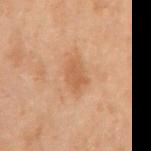| field | value |
|---|---|
| notes | imaged on a skin check; not biopsied |
| patient | male, approximately 70 years of age |
| image | ~15 mm crop, total-body skin-cancer survey |
| body site | the mid back |
| automated lesion analysis | a lesion color around L≈44 a*≈18 b*≈29 in CIELAB and roughly 6 lightness units darker than nearby skin; a border-irregularity rating of about 2.5/10, internal color variation of about 2 on a 0–10 scale, and a peripheral color-asymmetry measure near 0.5 |
| lighting | cross-polarized illumination |
| lesion size | about 3 mm |